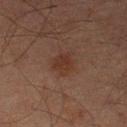This lesion was catalogued during total-body skin photography and was not selected for biopsy.